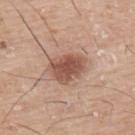This lesion was catalogued during total-body skin photography and was not selected for biopsy.
This is a white-light tile.
A male subject, aged 73–77.
A region of skin cropped from a whole-body photographic capture, roughly 15 mm wide.
Located on the upper back.